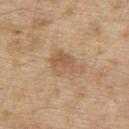{"biopsy_status": "not biopsied; imaged during a skin examination", "patient": {"sex": "male", "age_approx": 70}, "lighting": "white-light", "image": {"source": "total-body photography crop", "field_of_view_mm": 15}, "site": "upper back", "lesion_size": {"long_diameter_mm_approx": 4.5}}